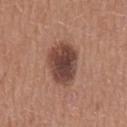The lesion was tiled from a total-body skin photograph and was not biopsied.
A male patient, aged 63 to 67.
Imaged with white-light lighting.
The recorded lesion diameter is about 5.5 mm.
Located on the mid back.
Cropped from a whole-body photographic skin survey; the tile spans about 15 mm.
Automated image analysis of the tile measured a lesion area of about 15 mm², an eccentricity of roughly 0.75, and a shape-asymmetry score of about 0.15 (0 = symmetric). It also reported a lesion–skin lightness drop of about 15 and a lesion-to-skin contrast of about 11 (normalized; higher = more distinct).